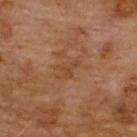| feature | finding |
|---|---|
| notes | no biopsy performed (imaged during a skin exam) |
| diameter | ~3 mm (longest diameter) |
| patient | male, aged 58 to 62 |
| imaging modality | total-body-photography crop, ~15 mm field of view |
| anatomic site | the front of the torso |
| image-analysis metrics | a shape eccentricity near 0.75 and two-axis asymmetry of about 0.7; a border-irregularity rating of about 7/10, a within-lesion color-variation index near 0/10, and radial color variation of about 0 |
| lighting | cross-polarized |